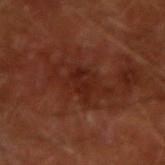Background: The lesion is located on the right forearm. Cropped from a total-body skin-imaging series; the visible field is about 15 mm. About 3 mm across. The subject is a male roughly 65 years of age.